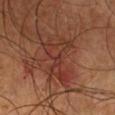{"biopsy_status": "not biopsied; imaged during a skin examination", "image": {"source": "total-body photography crop", "field_of_view_mm": 15}, "patient": {"sex": "male", "age_approx": 40}, "site": "chest"}